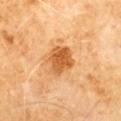The lesion was tiled from a total-body skin photograph and was not biopsied.
The subject is a male about 60 years old.
About 3.5 mm across.
Captured under cross-polarized illumination.
The lesion is located on the chest.
Cropped from a whole-body photographic skin survey; the tile spans about 15 mm.